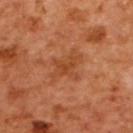follow-up: catalogued during a skin exam; not biopsied | patient: female, roughly 55 years of age | image: 15 mm crop, total-body photography | anatomic site: the upper back.